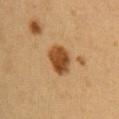Impression:
The lesion was photographed on a routine skin check and not biopsied; there is no pathology result.